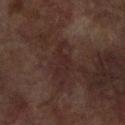Captured during whole-body skin photography for melanoma surveillance; the lesion was not biopsied. A female patient, about 80 years old. A 15 mm crop from a total-body photograph taken for skin-cancer surveillance. From the arm.